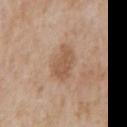Impression:
The lesion was photographed on a routine skin check and not biopsied; there is no pathology result.
Context:
Located on the front of the torso. A male patient, aged around 65. A region of skin cropped from a whole-body photographic capture, roughly 15 mm wide. Automated image analysis of the tile measured a lesion area of about 10 mm², a shape eccentricity near 0.75, and a symmetry-axis asymmetry near 0.2. It also reported border irregularity of about 2 on a 0–10 scale. The recorded lesion diameter is about 4 mm.